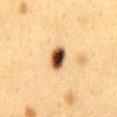biopsy status: total-body-photography surveillance lesion; no biopsy | location: the mid back | lesion size: about 3 mm | lighting: cross-polarized | patient: female, aged 38–42 | acquisition: 15 mm crop, total-body photography | TBP lesion metrics: a lesion color around L≈42 a*≈17 b*≈32 in CIELAB, about 24 CIELAB-L* units darker than the surrounding skin, and a normalized border contrast of about 16.5; a border-irregularity index near 1.5/10 and internal color variation of about 10 on a 0–10 scale; lesion-presence confidence of about 100/100.